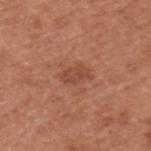Case summary:
– workup · catalogued during a skin exam; not biopsied
– image-analysis metrics · a shape eccentricity near 0.85 and a symmetry-axis asymmetry near 0.2; a lesion color around L≈47 a*≈25 b*≈31 in CIELAB and a lesion–skin lightness drop of about 8
– image · 15 mm crop, total-body photography
– patient · male, aged approximately 55
– size · ~3.5 mm (longest diameter)
– tile lighting · white-light illumination
– anatomic site · the upper back